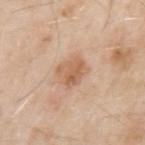biopsy status = total-body-photography surveillance lesion; no biopsy | patient = male, aged 53–57 | lesion size = ~3.5 mm (longest diameter) | illumination = white-light | image-analysis metrics = an area of roughly 7.5 mm² and two-axis asymmetry of about 0.25 | image = 15 mm crop, total-body photography | location = the arm.